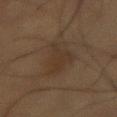biopsy_status: not biopsied; imaged during a skin examination
patient:
  sex: male
  age_approx: 55
lighting: cross-polarized
image:
  source: total-body photography crop
  field_of_view_mm: 15
lesion_size:
  long_diameter_mm_approx: 6.0
site: abdomen